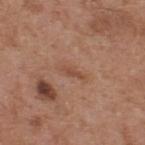Assessment: The lesion was photographed on a routine skin check and not biopsied; there is no pathology result. Image and clinical context: Longest diameter approximately 3 mm. Located on the upper back. A region of skin cropped from a whole-body photographic capture, roughly 15 mm wide. A male patient aged approximately 55. Imaged with white-light lighting.